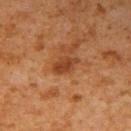| key | value |
|---|---|
| notes | total-body-photography surveillance lesion; no biopsy |
| image source | 15 mm crop, total-body photography |
| patient | male, about 60 years old |
| TBP lesion metrics | a footprint of about 5 mm² and two-axis asymmetry of about 0.3; a border-irregularity rating of about 3/10, a color-variation rating of about 2/10, and radial color variation of about 0.5 |
| lesion size | ≈3.5 mm |
| anatomic site | the arm |
| tile lighting | cross-polarized illumination |